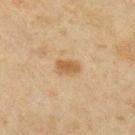Background:
The lesion is on the arm. Imaged with cross-polarized lighting. Cropped from a whole-body photographic skin survey; the tile spans about 15 mm. A female subject in their mid-40s. Automated image analysis of the tile measured a border-irregularity index near 2/10, a color-variation rating of about 1.5/10, and a peripheral color-asymmetry measure near 0.5.